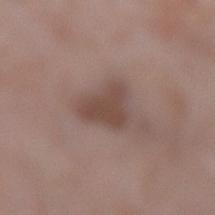Q: Is there a histopathology result?
A: no biopsy performed (imaged during a skin exam)
Q: Where on the body is the lesion?
A: the left lower leg
Q: What are the patient's age and sex?
A: female, roughly 70 years of age
Q: What is the imaging modality?
A: 15 mm crop, total-body photography